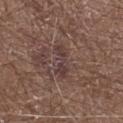Imaged during a routine full-body skin examination; the lesion was not biopsied and no histopathology is available. Cropped from a total-body skin-imaging series; the visible field is about 15 mm. The lesion is located on the left forearm. A male patient aged approximately 60. Imaged with white-light lighting. About 4.5 mm across.